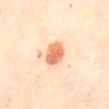{
  "biopsy_status": "not biopsied; imaged during a skin examination",
  "site": "abdomen",
  "automated_metrics": {
    "eccentricity": 0.4,
    "shape_asymmetry": 0.1,
    "cielab_L": 73,
    "cielab_a": 25,
    "cielab_b": 39,
    "vs_skin_darker_L": 15.0,
    "vs_skin_contrast_norm": 9.0,
    "border_irregularity_0_10": 1.0,
    "color_variation_0_10": 4.0,
    "peripheral_color_asymmetry": 1.0
  },
  "patient": {
    "sex": "female",
    "age_approx": 60
  },
  "lesion_size": {
    "long_diameter_mm_approx": 3.0
  },
  "lighting": "cross-polarized",
  "image": {
    "source": "total-body photography crop",
    "field_of_view_mm": 15
  }
}